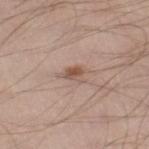Clinical impression: The lesion was tiled from a total-body skin photograph and was not biopsied. Context: The patient is a male roughly 35 years of age. The lesion's longest dimension is about 2.5 mm. On the leg. The lesion-visualizer software estimated a lesion area of about 3.5 mm² and an eccentricity of roughly 0.7. The analysis additionally found a within-lesion color-variation index near 3/10 and a peripheral color-asymmetry measure near 1. A roughly 15 mm field-of-view crop from a total-body skin photograph.